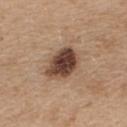biopsy status = total-body-photography surveillance lesion; no biopsy
patient = female, roughly 45 years of age
site = the upper back
tile lighting = white-light
size = about 4.5 mm
imaging modality = 15 mm crop, total-body photography